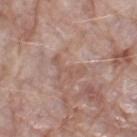biopsy status: no biopsy performed (imaged during a skin exam); body site: the leg; imaging modality: ~15 mm crop, total-body skin-cancer survey; lesion size: about 4.5 mm; subject: female, aged approximately 70.